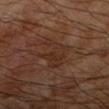<lesion>
<biopsy_status>not biopsied; imaged during a skin examination</biopsy_status>
<lesion_size>
  <long_diameter_mm_approx>4.5</long_diameter_mm_approx>
</lesion_size>
<patient>
  <sex>male</sex>
  <age_approx>70</age_approx>
</patient>
<lighting>cross-polarized</lighting>
<site>right forearm</site>
<image>
  <source>total-body photography crop</source>
  <field_of_view_mm>15</field_of_view_mm>
</image>
</lesion>